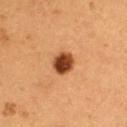Imaged during a routine full-body skin examination; the lesion was not biopsied and no histopathology is available. Located on the left upper arm. Longest diameter approximately 3 mm. A male subject, aged 48 to 52. The tile uses cross-polarized illumination. A 15 mm crop from a total-body photograph taken for skin-cancer surveillance.